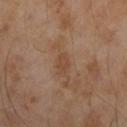The subject is a male in their mid- to late 50s. The lesion is on the left upper arm. A 15 mm close-up extracted from a 3D total-body photography capture.